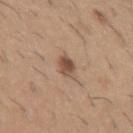| key | value |
|---|---|
| workup | no biopsy performed (imaged during a skin exam) |
| imaging modality | 15 mm crop, total-body photography |
| subject | male, about 60 years old |
| size | about 3 mm |
| body site | the left upper arm |
| automated lesion analysis | a lesion area of about 4.5 mm² and a shape-asymmetry score of about 0.2 (0 = symmetric); a within-lesion color-variation index near 5.5/10 and peripheral color asymmetry of about 2 |